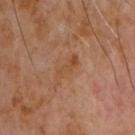The lesion was photographed on a routine skin check and not biopsied; there is no pathology result.
A male patient, about 60 years old.
A close-up tile cropped from a whole-body skin photograph, about 15 mm across.
The lesion is on the chest.
This is a cross-polarized tile.
The lesion's longest dimension is about 4 mm.
The lesion-visualizer software estimated a lesion color around L≈44 a*≈20 b*≈32 in CIELAB, a lesion–skin lightness drop of about 5, and a normalized lesion–skin contrast near 5.5. The software also gave a lesion-detection confidence of about 100/100.